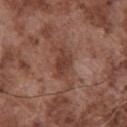| key | value |
|---|---|
| biopsy status | imaged on a skin check; not biopsied |
| patient | male, aged approximately 75 |
| image | total-body-photography crop, ~15 mm field of view |
| diameter | about 3.5 mm |
| TBP lesion metrics | a within-lesion color-variation index near 2/10 and a peripheral color-asymmetry measure near 0.5; a classifier nevus-likeness of about 15/100 |
| lighting | white-light illumination |
| location | the chest |